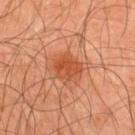automated metrics: an outline eccentricity of about 0.5 (0 = round, 1 = elongated) and a symmetry-axis asymmetry near 0.2; a border-irregularity index near 2/10 and a color-variation rating of about 3/10; an automated nevus-likeness rating near 90 out of 100
subject: male, aged approximately 45
site: the mid back
size: about 3.5 mm
lighting: cross-polarized
imaging modality: ~15 mm tile from a whole-body skin photo